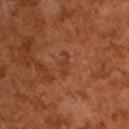Q: Is there a histopathology result?
A: total-body-photography surveillance lesion; no biopsy
Q: How was the tile lit?
A: cross-polarized illumination
Q: Patient demographics?
A: male, aged 63–67
Q: How was this image acquired?
A: ~15 mm tile from a whole-body skin photo
Q: Automated lesion metrics?
A: a mean CIELAB color near L≈38 a*≈25 b*≈33, a lesion–skin lightness drop of about 5, and a normalized lesion–skin contrast near 5
Q: What is the lesion's diameter?
A: ≈3 mm